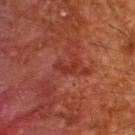Assessment: Recorded during total-body skin imaging; not selected for excision or biopsy. Clinical summary: Imaged with cross-polarized lighting. Cropped from a total-body skin-imaging series; the visible field is about 15 mm. A male subject in their 60s. The lesion is on the upper back. The recorded lesion diameter is about 3 mm. The lesion-visualizer software estimated a mean CIELAB color near L≈26 a*≈26 b*≈24, about 5 CIELAB-L* units darker than the surrounding skin, and a lesion-to-skin contrast of about 6 (normalized; higher = more distinct). The analysis additionally found a lesion-detection confidence of about 80/100.